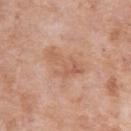{"biopsy_status": "not biopsied; imaged during a skin examination", "patient": {"sex": "female", "age_approx": 70}, "site": "left upper arm", "image": {"source": "total-body photography crop", "field_of_view_mm": 15}}